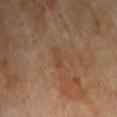| feature | finding |
|---|---|
| biopsy status | catalogued during a skin exam; not biopsied |
| site | the chest |
| image | 15 mm crop, total-body photography |
| patient | male, in their 70s |
| illumination | cross-polarized |
| lesion size | ≈3 mm |